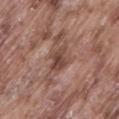Clinical summary: A lesion tile, about 15 mm wide, cut from a 3D total-body photograph. The subject is a male in their mid-70s. Located on the front of the torso.